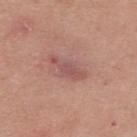{
  "biopsy_status": "not biopsied; imaged during a skin examination",
  "image": {
    "source": "total-body photography crop",
    "field_of_view_mm": 15
  },
  "automated_metrics": {
    "area_mm2_approx": 6.0,
    "shape_asymmetry": 0.3,
    "border_irregularity_0_10": 4.0,
    "color_variation_0_10": 1.5,
    "peripheral_color_asymmetry": 0.5
  },
  "site": "back",
  "lesion_size": {
    "long_diameter_mm_approx": 4.5
  },
  "patient": {
    "sex": "male",
    "age_approx": 25
  }
}